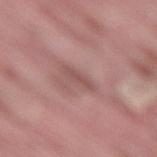From the lower back. A close-up tile cropped from a whole-body skin photograph, about 15 mm across. A male subject, approximately 40 years of age. Captured under white-light illumination.